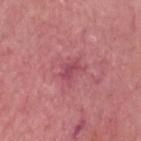Case summary:
* follow-up: catalogued during a skin exam; not biopsied
* subject: male, aged around 65
* illumination: white-light illumination
* lesion diameter: ~3 mm (longest diameter)
* image: ~15 mm tile from a whole-body skin photo
* location: the head or neck